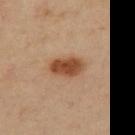No biopsy was performed on this lesion — it was imaged during a full skin examination and was not determined to be concerning.